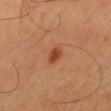{
  "biopsy_status": "not biopsied; imaged during a skin examination",
  "site": "mid back",
  "patient": {
    "sex": "male",
    "age_approx": 65
  },
  "image": {
    "source": "total-body photography crop",
    "field_of_view_mm": 15
  },
  "lesion_size": {
    "long_diameter_mm_approx": 2.0
  },
  "automated_metrics": {
    "cielab_L": 44,
    "cielab_a": 27,
    "cielab_b": 35,
    "vs_skin_darker_L": 10.0,
    "vs_skin_contrast_norm": 8.0,
    "color_variation_0_10": 2.0,
    "peripheral_color_asymmetry": 0.5,
    "lesion_detection_confidence_0_100": 100
  },
  "lighting": "cross-polarized"
}